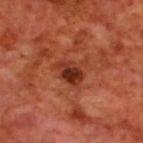Impression: This lesion was catalogued during total-body skin photography and was not selected for biopsy.